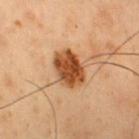Assessment: This lesion was catalogued during total-body skin photography and was not selected for biopsy. Acquisition and patient details: The lesion is located on the chest. Measured at roughly 4 mm in maximum diameter. The tile uses cross-polarized illumination. The subject is a male aged 53–57. A region of skin cropped from a whole-body photographic capture, roughly 15 mm wide.